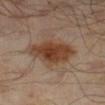No biopsy was performed on this lesion — it was imaged during a full skin examination and was not determined to be concerning.
An algorithmic analysis of the crop reported an area of roughly 16 mm² and a symmetry-axis asymmetry near 0.2. The software also gave a mean CIELAB color near L≈37 a*≈19 b*≈28, about 11 CIELAB-L* units darker than the surrounding skin, and a normalized border contrast of about 10.5. And it measured lesion-presence confidence of about 100/100.
Captured under cross-polarized illumination.
A 15 mm crop from a total-body photograph taken for skin-cancer surveillance.
The lesion is on the right lower leg.
The patient is a male aged approximately 65.